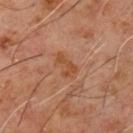{"patient": {"sex": "male", "age_approx": 60}, "automated_metrics": {"area_mm2_approx": 3.5, "eccentricity": 0.85, "shape_asymmetry": 0.5}, "site": "chest", "image": {"source": "total-body photography crop", "field_of_view_mm": 15}, "lesion_size": {"long_diameter_mm_approx": 3.0}, "lighting": "cross-polarized"}